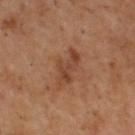<case>
  <biopsy_status>not biopsied; imaged during a skin examination</biopsy_status>
  <image>
    <source>total-body photography crop</source>
    <field_of_view_mm>15</field_of_view_mm>
  </image>
  <lighting>cross-polarized</lighting>
  <patient>
    <sex>male</sex>
    <age_approx>55</age_approx>
  </patient>
  <lesion_size>
    <long_diameter_mm_approx>4.5</long_diameter_mm_approx>
  </lesion_size>
  <site>upper back</site>
  <automated_metrics>
    <area_mm2_approx>7.5</area_mm2_approx>
    <eccentricity>0.85</eccentricity>
    <shape_asymmetry>0.4</shape_asymmetry>
    <vs_skin_darker_L>8.0</vs_skin_darker_L>
    <vs_skin_contrast_norm>6.5</vs_skin_contrast_norm>
    <color_variation_0_10>4.0</color_variation_0_10>
    <nevus_likeness_0_100>0</nevus_likeness_0_100>
    <lesion_detection_confidence_0_100>100</lesion_detection_confidence_0_100>
  </automated_metrics>
</case>